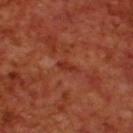* anatomic site: the back
* tile lighting: cross-polarized illumination
* image source: 15 mm crop, total-body photography
* patient: male, about 70 years old